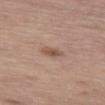Imaged during a routine full-body skin examination; the lesion was not biopsied and no histopathology is available.
The tile uses white-light illumination.
A female patient, about 65 years old.
Automated tile analysis of the lesion measured a footprint of about 3.5 mm², an outline eccentricity of about 0.85 (0 = round, 1 = elongated), and a symmetry-axis asymmetry near 0.2. It also reported a nevus-likeness score of about 65/100 and lesion-presence confidence of about 100/100.
A 15 mm close-up tile from a total-body photography series done for melanoma screening.
The lesion is located on the left thigh.
Measured at roughly 3 mm in maximum diameter.